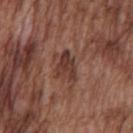Clinical impression: No biopsy was performed on this lesion — it was imaged during a full skin examination and was not determined to be concerning. Context: The subject is a male in their mid-70s. The lesion is located on the chest. Approximately 3.5 mm at its widest. The tile uses white-light illumination. A 15 mm close-up extracted from a 3D total-body photography capture. An algorithmic analysis of the crop reported a lesion area of about 5.5 mm², a shape eccentricity near 0.75, and a symmetry-axis asymmetry near 0.45. It also reported about 9 CIELAB-L* units darker than the surrounding skin and a lesion-to-skin contrast of about 8 (normalized; higher = more distinct). The software also gave a border-irregularity index near 5/10, a within-lesion color-variation index near 2.5/10, and radial color variation of about 1. And it measured a nevus-likeness score of about 0/100 and a detector confidence of about 95 out of 100 that the crop contains a lesion.